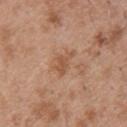Q: Is there a histopathology result?
A: catalogued during a skin exam; not biopsied
Q: Who is the patient?
A: male, aged approximately 50
Q: Lesion location?
A: the upper back
Q: What kind of image is this?
A: 15 mm crop, total-body photography
Q: What is the lesion's diameter?
A: about 3.5 mm